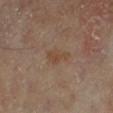<case>
<biopsy_status>not biopsied; imaged during a skin examination</biopsy_status>
<site>leg</site>
<patient>
  <sex>male</sex>
  <age_approx>65</age_approx>
</patient>
<lesion_size>
  <long_diameter_mm_approx>3.0</long_diameter_mm_approx>
</lesion_size>
<image>
  <source>total-body photography crop</source>
  <field_of_view_mm>15</field_of_view_mm>
</image>
<automated_metrics>
  <area_mm2_approx>3.5</area_mm2_approx>
  <shape_asymmetry>0.35</shape_asymmetry>
  <cielab_L>44</cielab_L>
  <cielab_a>17</cielab_a>
  <cielab_b>29</cielab_b>
  <vs_skin_darker_L>6.0</vs_skin_darker_L>
  <vs_skin_contrast_norm>6.5</vs_skin_contrast_norm>
  <border_irregularity_0_10>4.0</border_irregularity_0_10>
  <peripheral_color_asymmetry>0.5</peripheral_color_asymmetry>
</automated_metrics>
</case>